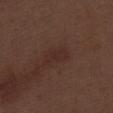– workup · no biopsy performed (imaged during a skin exam)
– acquisition · 15 mm crop, total-body photography
– site · the right thigh
– lesion size · ≈3 mm
– automated metrics · an area of roughly 3.5 mm², a shape eccentricity near 0.9, and a symmetry-axis asymmetry near 0.45; a border-irregularity rating of about 4.5/10; an automated nevus-likeness rating near 5 out of 100
– tile lighting · white-light
– subject · male, approximately 70 years of age